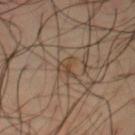<tbp_lesion>
<biopsy_status>not biopsied; imaged during a skin examination</biopsy_status>
<lesion_size>
  <long_diameter_mm_approx>2.5</long_diameter_mm_approx>
</lesion_size>
<site>chest</site>
<lighting>cross-polarized</lighting>
<image>
  <source>total-body photography crop</source>
  <field_of_view_mm>15</field_of_view_mm>
</image>
<patient>
  <sex>male</sex>
  <age_approx>65</age_approx>
</patient>
</tbp_lesion>